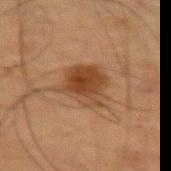automated_metrics:
  nevus_likeness_0_100: 90
  lesion_detection_confidence_0_100: 100
patient:
  sex: male
  age_approx: 75
site: leg
lighting: cross-polarized
lesion_size:
  long_diameter_mm_approx: 5.0
image:
  source: total-body photography crop
  field_of_view_mm: 15